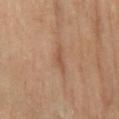Part of a total-body skin-imaging series; this lesion was reviewed on a skin check and was not flagged for biopsy.